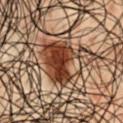{"lesion_size": {"long_diameter_mm_approx": 6.0}, "patient": {"sex": "male", "age_approx": 50}, "site": "chest", "image": {"source": "total-body photography crop", "field_of_view_mm": 15}, "lighting": "cross-polarized", "automated_metrics": {"area_mm2_approx": 20.0, "eccentricity": 0.75, "shape_asymmetry": 0.2, "cielab_L": 30, "cielab_a": 17, "cielab_b": 24, "vs_skin_darker_L": 11.0, "vs_skin_contrast_norm": 11.0}}